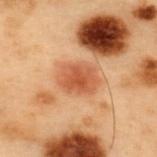The lesion was photographed on a routine skin check and not biopsied; there is no pathology result. A male patient in their mid- to late 50s. On the upper back. A lesion tile, about 15 mm wide, cut from a 3D total-body photograph. An algorithmic analysis of the crop reported a classifier nevus-likeness of about 10/100 and lesion-presence confidence of about 100/100. This is a cross-polarized tile. Measured at roughly 4.5 mm in maximum diameter.